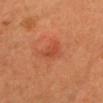notes: total-body-photography surveillance lesion; no biopsy
anatomic site: the head or neck
automated lesion analysis: a footprint of about 2.5 mm², an outline eccentricity of about 0.9 (0 = round, 1 = elongated), and a shape-asymmetry score of about 0.4 (0 = symmetric); a border-irregularity index near 3.5/10, a within-lesion color-variation index near 0/10, and radial color variation of about 0
image: total-body-photography crop, ~15 mm field of view
illumination: cross-polarized illumination
size: about 2.5 mm
subject: female, about 40 years old